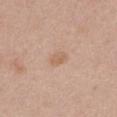workup = imaged on a skin check; not biopsied | lighting = white-light | subject = female, in their 40s | size = ≈2.5 mm | imaging modality = 15 mm crop, total-body photography | body site = the chest.